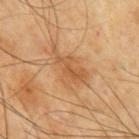Part of a total-body skin-imaging series; this lesion was reviewed on a skin check and was not flagged for biopsy.
The patient is a male in their mid-60s.
Located on the chest.
Cropped from a total-body skin-imaging series; the visible field is about 15 mm.